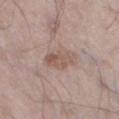Findings:
* notes — no biopsy performed (imaged during a skin exam)
* diameter — ~4 mm (longest diameter)
* image — ~15 mm tile from a whole-body skin photo
* body site — the left thigh
* illumination — white-light illumination
* subject — male, in their mid-60s